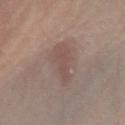Q: Was a biopsy performed?
A: catalogued during a skin exam; not biopsied
Q: What kind of image is this?
A: 15 mm crop, total-body photography
Q: Who is the patient?
A: female, aged 63 to 67
Q: What is the anatomic site?
A: the arm
Q: What lighting was used for the tile?
A: white-light illumination